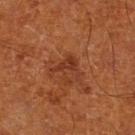workup: catalogued during a skin exam; not biopsied | location: the leg | imaging modality: total-body-photography crop, ~15 mm field of view | subject: male, approximately 65 years of age.